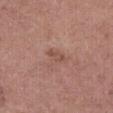<lesion>
  <biopsy_status>not biopsied; imaged during a skin examination</biopsy_status>
  <site>leg</site>
  <lesion_size>
    <long_diameter_mm_approx>2.5</long_diameter_mm_approx>
  </lesion_size>
  <lighting>white-light</lighting>
  <image>
    <source>total-body photography crop</source>
    <field_of_view_mm>15</field_of_view_mm>
  </image>
  <patient>
    <sex>female</sex>
    <age_approx>65</age_approx>
  </patient>
</lesion>